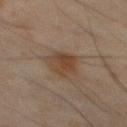Impression: This lesion was catalogued during total-body skin photography and was not selected for biopsy.